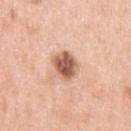illumination: white-light illumination | acquisition: 15 mm crop, total-body photography | lesion diameter: ~3.5 mm (longest diameter) | location: the left upper arm | patient: male, in their 60s | automated metrics: a lesion area of about 8 mm², a shape eccentricity near 0.5, and a shape-asymmetry score of about 0.15 (0 = symmetric); a mean CIELAB color near L≈59 a*≈23 b*≈31, roughly 17 lightness units darker than nearby skin, and a normalized lesion–skin contrast near 10.5; a within-lesion color-variation index near 5/10 and a peripheral color-asymmetry measure near 1.5.